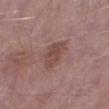{"biopsy_status": "not biopsied; imaged during a skin examination", "site": "left lower leg", "patient": {"sex": "male", "age_approx": 55}, "automated_metrics": {"area_mm2_approx": 7.0, "eccentricity": 0.8, "shape_asymmetry": 0.25, "cielab_L": 46, "cielab_a": 21, "cielab_b": 22, "lesion_detection_confidence_0_100": 100}, "lesion_size": {"long_diameter_mm_approx": 4.0}, "image": {"source": "total-body photography crop", "field_of_view_mm": 15}, "lighting": "white-light"}